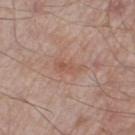| key | value |
|---|---|
| workup | imaged on a skin check; not biopsied |
| lighting | white-light illumination |
| image source | 15 mm crop, total-body photography |
| patient | male, roughly 55 years of age |
| size | about 4 mm |
| site | the right thigh |